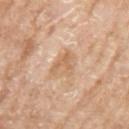workup = imaged on a skin check; not biopsied
patient = female, aged 73 to 77
imaging modality = ~15 mm tile from a whole-body skin photo
illumination = white-light
automated metrics = a normalized lesion–skin contrast near 6; border irregularity of about 6.5 on a 0–10 scale, a color-variation rating of about 2/10, and peripheral color asymmetry of about 0.5; a classifier nevus-likeness of about 0/100 and a detector confidence of about 100 out of 100 that the crop contains a lesion
location = the left upper arm